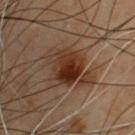Findings:
• patient — male, in their mid- to late 50s
• lesion diameter — ≈4.5 mm
• body site — the front of the torso
• acquisition — total-body-photography crop, ~15 mm field of view